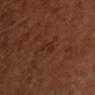About 2.5 mm across.
The lesion is located on the chest.
A male patient in their 50s.
A 15 mm close-up extracted from a 3D total-body photography capture.
Automated image analysis of the tile measured an area of roughly 2.5 mm², an outline eccentricity of about 0.85 (0 = round, 1 = elongated), and a shape-asymmetry score of about 0.45 (0 = symmetric). The analysis additionally found a lesion–skin lightness drop of about 5 and a normalized border contrast of about 5.5. The software also gave a color-variation rating of about 1/10 and a peripheral color-asymmetry measure near 0.5. The software also gave a nevus-likeness score of about 0/100 and lesion-presence confidence of about 100/100.